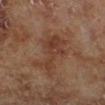| feature | finding |
|---|---|
| biopsy status | imaged on a skin check; not biopsied |
| anatomic site | the left lower leg |
| patient | male, aged approximately 70 |
| automated lesion analysis | a lesion color around L≈37 a*≈20 b*≈27 in CIELAB, about 7 CIELAB-L* units darker than the surrounding skin, and a normalized border contrast of about 6.5; a classifier nevus-likeness of about 0/100 and a lesion-detection confidence of about 100/100 |
| image source | ~15 mm crop, total-body skin-cancer survey |
| tile lighting | cross-polarized |
| lesion diameter | ~6.5 mm (longest diameter) |